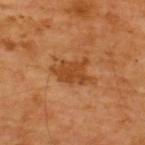location = the upper back; illumination = cross-polarized; image = total-body-photography crop, ~15 mm field of view; subject = male, aged around 65.